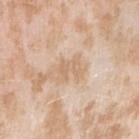Captured during whole-body skin photography for melanoma surveillance; the lesion was not biopsied.
Captured under white-light illumination.
The lesion is located on the right upper arm.
Longest diameter approximately 3 mm.
A 15 mm close-up tile from a total-body photography series done for melanoma screening.
A female patient approximately 25 years of age.